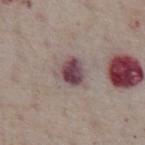Clinical impression:
The lesion was tiled from a total-body skin photograph and was not biopsied.
Clinical summary:
Imaged with white-light lighting. A close-up tile cropped from a whole-body skin photograph, about 15 mm across. Located on the abdomen. Measured at roughly 3 mm in maximum diameter. A male subject, aged around 75.